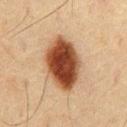Captured during whole-body skin photography for melanoma surveillance; the lesion was not biopsied.